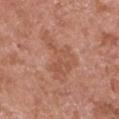A 15 mm close-up tile from a total-body photography series done for melanoma screening. A male subject, aged 63 to 67. From the left upper arm. Automated tile analysis of the lesion measured a lesion color around L≈54 a*≈24 b*≈31 in CIELAB and a lesion–skin lightness drop of about 6. The software also gave an automated nevus-likeness rating near 0 out of 100 and lesion-presence confidence of about 100/100. Captured under white-light illumination. About 6.5 mm across.